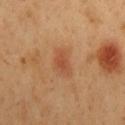Captured during whole-body skin photography for melanoma surveillance; the lesion was not biopsied.
Imaged with cross-polarized lighting.
Measured at roughly 3 mm in maximum diameter.
Located on the mid back.
Cropped from a total-body skin-imaging series; the visible field is about 15 mm.
An algorithmic analysis of the crop reported a lesion area of about 4 mm² and an eccentricity of roughly 0.85. And it measured radial color variation of about 0.5. The software also gave an automated nevus-likeness rating near 75 out of 100 and a lesion-detection confidence of about 100/100.
A male subject aged 48–52.